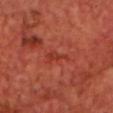No biopsy was performed on this lesion — it was imaged during a full skin examination and was not determined to be concerning.
From the chest.
The lesion's longest dimension is about 3 mm.
Cropped from a total-body skin-imaging series; the visible field is about 15 mm.
A male subject about 70 years old.